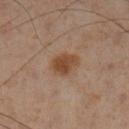biopsy_status: not biopsied; imaged during a skin examination
lighting: cross-polarized
lesion_size:
  long_diameter_mm_approx: 3.5
image:
  source: total-body photography crop
  field_of_view_mm: 15
site: left lower leg
patient:
  sex: male
  age_approx: 55